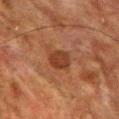follow-up = imaged on a skin check; not biopsied | site = the front of the torso | image source = ~15 mm crop, total-body skin-cancer survey | patient = male, in their mid- to late 60s.